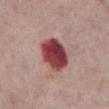Part of a total-body skin-imaging series; this lesion was reviewed on a skin check and was not flagged for biopsy.
The lesion is located on the abdomen.
The lesion's longest dimension is about 5 mm.
The subject is a male aged approximately 75.
This image is a 15 mm lesion crop taken from a total-body photograph.
Captured under white-light illumination.
Automated image analysis of the tile measured a lesion area of about 13 mm² and a shape eccentricity near 0.7. It also reported a border-irregularity index near 1.5/10, a within-lesion color-variation index near 6/10, and radial color variation of about 2. And it measured a nevus-likeness score of about 0/100 and lesion-presence confidence of about 100/100.